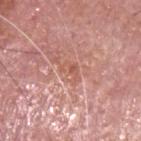Captured during whole-body skin photography for melanoma surveillance; the lesion was not biopsied.
A male subject, approximately 75 years of age.
The lesion is located on the head or neck.
Imaged with white-light lighting.
Cropped from a whole-body photographic skin survey; the tile spans about 15 mm.
The lesion's longest dimension is about 2.5 mm.
The total-body-photography lesion software estimated a mean CIELAB color near L≈57 a*≈25 b*≈29, roughly 7 lightness units darker than nearby skin, and a normalized border contrast of about 5.5. And it measured a border-irregularity index near 5.5/10 and peripheral color asymmetry of about 1. The software also gave a nevus-likeness score of about 0/100.